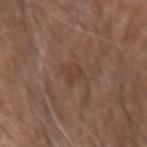The lesion was tiled from a total-body skin photograph and was not biopsied. A 15 mm crop from a total-body photograph taken for skin-cancer surveillance. Longest diameter approximately 2.5 mm. The patient is a male aged 68 to 72. Automated image analysis of the tile measured an average lesion color of about L≈40 a*≈18 b*≈26 (CIELAB), about 5 CIELAB-L* units darker than the surrounding skin, and a lesion-to-skin contrast of about 5 (normalized; higher = more distinct). The lesion is on the right forearm. Captured under white-light illumination.